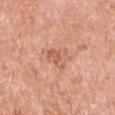- biopsy status — total-body-photography surveillance lesion; no biopsy
- location — the right upper arm
- lighting — white-light
- imaging modality — 15 mm crop, total-body photography
- TBP lesion metrics — an area of roughly 6.5 mm², an eccentricity of roughly 0.5, and two-axis asymmetry of about 0.45; a border-irregularity index near 4.5/10 and internal color variation of about 4 on a 0–10 scale
- lesion diameter — about 3 mm
- patient — male, aged 68 to 72